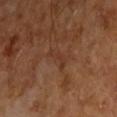{"biopsy_status": "not biopsied; imaged during a skin examination", "lighting": "cross-polarized", "patient": {"sex": "male", "age_approx": 65}, "site": "right upper arm", "lesion_size": {"long_diameter_mm_approx": 3.5}, "image": {"source": "total-body photography crop", "field_of_view_mm": 15}}